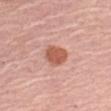No biopsy was performed on this lesion — it was imaged during a full skin examination and was not determined to be concerning.
The lesion is on the left upper arm.
Automated tile analysis of the lesion measured an area of roughly 7 mm², an outline eccentricity of about 0.75 (0 = round, 1 = elongated), and a shape-asymmetry score of about 0.2 (0 = symmetric). And it measured an automated nevus-likeness rating near 95 out of 100 and lesion-presence confidence of about 100/100.
The tile uses white-light illumination.
A 15 mm crop from a total-body photograph taken for skin-cancer surveillance.
A female subject, in their mid- to late 60s.
The lesion's longest dimension is about 3.5 mm.